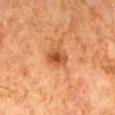Notes:
- biopsy status · total-body-photography surveillance lesion; no biopsy
- body site · the abdomen
- automated metrics · a footprint of about 5 mm², an eccentricity of roughly 0.75, and two-axis asymmetry of about 0.2; a lesion-to-skin contrast of about 8 (normalized; higher = more distinct)
- subject · male, aged 78 to 82
- size · ≈3 mm
- image source · total-body-photography crop, ~15 mm field of view
- illumination · cross-polarized